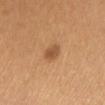{"biopsy_status": "not biopsied; imaged during a skin examination", "patient": {"sex": "female", "age_approx": 40}, "lesion_size": {"long_diameter_mm_approx": 2.5}, "site": "left lower leg", "image": {"source": "total-body photography crop", "field_of_view_mm": 15}}